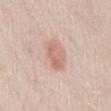<lesion>
<biopsy_status>not biopsied; imaged during a skin examination</biopsy_status>
<site>abdomen</site>
<patient>
  <sex>male</sex>
  <age_approx>25</age_approx>
</patient>
<image>
  <source>total-body photography crop</source>
  <field_of_view_mm>15</field_of_view_mm>
</image>
</lesion>